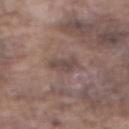{
  "biopsy_status": "not biopsied; imaged during a skin examination",
  "patient": {
    "sex": "male",
    "age_approx": 75
  },
  "automated_metrics": {
    "area_mm2_approx": 4.0,
    "eccentricity": 0.7,
    "shape_asymmetry": 0.35,
    "cielab_L": 45,
    "cielab_a": 15,
    "cielab_b": 19,
    "vs_skin_darker_L": 8.0,
    "vs_skin_contrast_norm": 6.5
  },
  "lighting": "white-light",
  "lesion_size": {
    "long_diameter_mm_approx": 2.5
  },
  "image": {
    "source": "total-body photography crop",
    "field_of_view_mm": 15
  },
  "site": "abdomen"
}